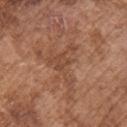Impression: This lesion was catalogued during total-body skin photography and was not selected for biopsy. Clinical summary: A male subject, about 75 years old. The lesion's longest dimension is about 5.5 mm. The lesion is on the front of the torso. This image is a 15 mm lesion crop taken from a total-body photograph.